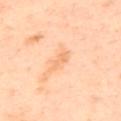biopsy status: total-body-photography surveillance lesion; no biopsy
patient: female, in their mid-50s
body site: the upper back
size: ≈3.5 mm
lighting: cross-polarized
acquisition: ~15 mm crop, total-body skin-cancer survey
automated lesion analysis: a lesion-to-skin contrast of about 5 (normalized; higher = more distinct)